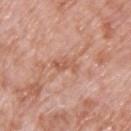workup: catalogued during a skin exam; not biopsied | image-analysis metrics: a within-lesion color-variation index near 0/10 and radial color variation of about 0 | subject: male, approximately 70 years of age | image: 15 mm crop, total-body photography | size: about 3.5 mm | anatomic site: the upper back | lighting: white-light.